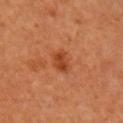Acquisition and patient details:
The total-body-photography lesion software estimated a lesion area of about 5 mm². It also reported a mean CIELAB color near L≈43 a*≈29 b*≈38, roughly 9 lightness units darker than nearby skin, and a lesion-to-skin contrast of about 7 (normalized; higher = more distinct). The lesion is located on the right upper arm. Captured under cross-polarized illumination. A male patient, aged 58 to 62. Cropped from a whole-body photographic skin survey; the tile spans about 15 mm.